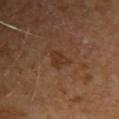Captured during whole-body skin photography for melanoma surveillance; the lesion was not biopsied. A female subject, roughly 65 years of age. A region of skin cropped from a whole-body photographic capture, roughly 15 mm wide. Imaged with cross-polarized lighting. Longest diameter approximately 3.5 mm. An algorithmic analysis of the crop reported a lesion area of about 4.5 mm², a shape eccentricity near 0.8, and two-axis asymmetry of about 0.5. The analysis additionally found an average lesion color of about L≈33 a*≈19 b*≈30 (CIELAB), about 6 CIELAB-L* units darker than the surrounding skin, and a normalized border contrast of about 6. And it measured a border-irregularity rating of about 5/10, a within-lesion color-variation index near 1.5/10, and peripheral color asymmetry of about 0.5. Located on the chest.